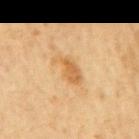biopsy_status: not biopsied; imaged during a skin examination
lighting: cross-polarized
lesion_size:
  long_diameter_mm_approx: 4.5
image:
  source: total-body photography crop
  field_of_view_mm: 15
automated_metrics:
  eccentricity: 0.9
  shape_asymmetry: 0.3
  color_variation_0_10: 2.0
patient:
  sex: male
  age_approx: 60
site: mid back